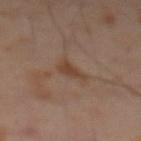biopsy status: no biopsy performed (imaged during a skin exam)
subject: male, about 65 years old
automated metrics: a lesion color around L≈40 a*≈17 b*≈27 in CIELAB, a lesion–skin lightness drop of about 7, and a normalized lesion–skin contrast near 7; a border-irregularity rating of about 4/10, a color-variation rating of about 2/10, and peripheral color asymmetry of about 0.5; an automated nevus-likeness rating near 0 out of 100 and lesion-presence confidence of about 100/100
illumination: cross-polarized
image: ~15 mm crop, total-body skin-cancer survey
lesion diameter: about 3 mm